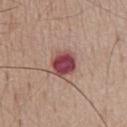Q: Was a biopsy performed?
A: total-body-photography surveillance lesion; no biopsy
Q: What is the lesion's diameter?
A: ~3.5 mm (longest diameter)
Q: Patient demographics?
A: male, aged around 55
Q: Illumination type?
A: white-light
Q: What is the imaging modality?
A: 15 mm crop, total-body photography